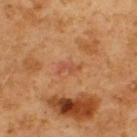Clinical impression: The lesion was tiled from a total-body skin photograph and was not biopsied. Clinical summary: The total-body-photography lesion software estimated a border-irregularity index near 6/10, a within-lesion color-variation index near 0/10, and a peripheral color-asymmetry measure near 0. A 15 mm close-up tile from a total-body photography series done for melanoma screening. On the back. A male patient, aged around 60. About 2.5 mm across.